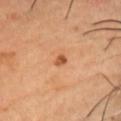Assessment:
Imaged during a routine full-body skin examination; the lesion was not biopsied and no histopathology is available.
Acquisition and patient details:
The subject is a male aged approximately 55. The lesion-visualizer software estimated an average lesion color of about L≈55 a*≈27 b*≈40 (CIELAB), a lesion–skin lightness drop of about 12, and a normalized border contrast of about 8.5. It also reported a border-irregularity index near 2.5/10 and a peripheral color-asymmetry measure near 1. The analysis additionally found an automated nevus-likeness rating near 65 out of 100 and lesion-presence confidence of about 100/100. Longest diameter approximately 1.5 mm. A close-up tile cropped from a whole-body skin photograph, about 15 mm across. The lesion is located on the head or neck.